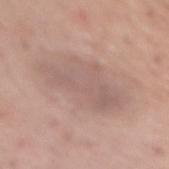{"biopsy_status": "not biopsied; imaged during a skin examination", "image": {"source": "total-body photography crop", "field_of_view_mm": 15}, "site": "mid back", "lighting": "white-light", "patient": {"sex": "female", "age_approx": 65}}